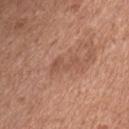image = ~15 mm tile from a whole-body skin photo; subject = male, in their mid- to late 50s; lesion size = ~3.5 mm (longest diameter); anatomic site = the chest; tile lighting = white-light illumination.